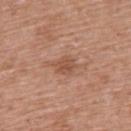Impression: Part of a total-body skin-imaging series; this lesion was reviewed on a skin check and was not flagged for biopsy. Image and clinical context: The lesion is located on the upper back. Measured at roughly 2.5 mm in maximum diameter. Cropped from a whole-body photographic skin survey; the tile spans about 15 mm. Captured under white-light illumination. Automated tile analysis of the lesion measured an average lesion color of about L≈52 a*≈22 b*≈31 (CIELAB), a lesion–skin lightness drop of about 8, and a normalized border contrast of about 6. The analysis additionally found a border-irregularity rating of about 2.5/10, a within-lesion color-variation index near 2.5/10, and radial color variation of about 1. The analysis additionally found an automated nevus-likeness rating near 0 out of 100. A female patient aged approximately 60.